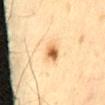Q: Was a biopsy performed?
A: total-body-photography surveillance lesion; no biopsy
Q: Automated lesion metrics?
A: a lesion color around L≈56 a*≈18 b*≈38 in CIELAB and roughly 14 lightness units darker than nearby skin; a border-irregularity rating of about 1.5/10 and internal color variation of about 6.5 on a 0–10 scale
Q: How was the tile lit?
A: cross-polarized
Q: What is the anatomic site?
A: the lower back
Q: How was this image acquired?
A: total-body-photography crop, ~15 mm field of view
Q: What are the patient's age and sex?
A: male, aged 58 to 62